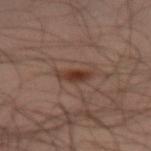biopsy_status: not biopsied; imaged during a skin examination
lighting: cross-polarized
image:
  source: total-body photography crop
  field_of_view_mm: 15
site: leg
patient:
  sex: male
  age_approx: 45
lesion_size:
  long_diameter_mm_approx: 3.5